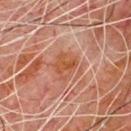This lesion was catalogued during total-body skin photography and was not selected for biopsy. The lesion-visualizer software estimated a mean CIELAB color near L≈40 a*≈21 b*≈29 and a lesion-to-skin contrast of about 7.5 (normalized; higher = more distinct). And it measured an automated nevus-likeness rating near 0 out of 100. A male subject, aged 78 to 82. From the chest. A 15 mm crop from a total-body photograph taken for skin-cancer surveillance. Longest diameter approximately 3.5 mm.